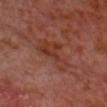workup: imaged on a skin check; not biopsied
patient: male, roughly 55 years of age
TBP lesion metrics: a footprint of about 11 mm², a shape eccentricity near 0.8, and a symmetry-axis asymmetry near 0.5; border irregularity of about 6 on a 0–10 scale, a within-lesion color-variation index near 4/10, and peripheral color asymmetry of about 1
diameter: about 5 mm
illumination: cross-polarized illumination
acquisition: total-body-photography crop, ~15 mm field of view
site: the head or neck